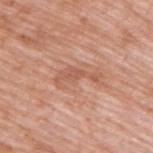Impression: Captured during whole-body skin photography for melanoma surveillance; the lesion was not biopsied.